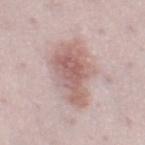<tbp_lesion>
<biopsy_status>not biopsied; imaged during a skin examination</biopsy_status>
<automated_metrics>
  <area_mm2_approx>21.0</area_mm2_approx>
  <eccentricity>0.85</eccentricity>
  <shape_asymmetry>0.3</shape_asymmetry>
  <border_irregularity_0_10>4.0</border_irregularity_0_10>
  <color_variation_0_10>4.5</color_variation_0_10>
  <peripheral_color_asymmetry>1.5</peripheral_color_asymmetry>
</automated_metrics>
<site>right thigh</site>
<patient>
  <sex>female</sex>
  <age_approx>30</age_approx>
</patient>
<lighting>white-light</lighting>
<lesion_size>
  <long_diameter_mm_approx>7.5</long_diameter_mm_approx>
</lesion_size>
<image>
  <source>total-body photography crop</source>
  <field_of_view_mm>15</field_of_view_mm>
</image>
</tbp_lesion>